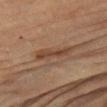Q: Was a biopsy performed?
A: catalogued during a skin exam; not biopsied
Q: What is the imaging modality?
A: total-body-photography crop, ~15 mm field of view
Q: What are the patient's age and sex?
A: female, about 80 years old
Q: What lighting was used for the tile?
A: cross-polarized illumination
Q: Where on the body is the lesion?
A: the left upper arm
Q: Lesion size?
A: ~5 mm (longest diameter)